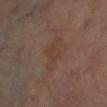notes=catalogued during a skin exam; not biopsied
imaging modality=~15 mm tile from a whole-body skin photo
body site=the right leg
lighting=cross-polarized
lesion diameter=≈4.5 mm
subject=female, aged 58–62
automated metrics=a shape eccentricity near 0.85 and a symmetry-axis asymmetry near 0.3; a lesion color around L≈39 a*≈17 b*≈26 in CIELAB and a normalized lesion–skin contrast near 5; border irregularity of about 3.5 on a 0–10 scale and internal color variation of about 1.5 on a 0–10 scale; an automated nevus-likeness rating near 0 out of 100